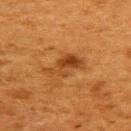• biopsy status — imaged on a skin check; not biopsied
• size — ≈5 mm
• imaging modality — ~15 mm tile from a whole-body skin photo
• site — the upper back
• patient — female, about 50 years old
• lighting — cross-polarized